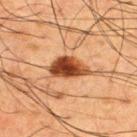Captured under cross-polarized illumination.
A male patient, aged approximately 60.
On the upper back.
Approximately 5 mm at its widest.
A 15 mm close-up tile from a total-body photography series done for melanoma screening.
The total-body-photography lesion software estimated a border-irregularity rating of about 2.5/10 and internal color variation of about 7 on a 0–10 scale. And it measured a classifier nevus-likeness of about 100/100 and a lesion-detection confidence of about 100/100.